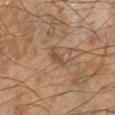Impression: This lesion was catalogued during total-body skin photography and was not selected for biopsy. Background: A lesion tile, about 15 mm wide, cut from a 3D total-body photograph. Captured under cross-polarized illumination. Located on the leg. A male subject, aged 43–47.